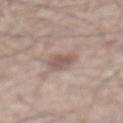notes = imaged on a skin check; not biopsied
image = total-body-photography crop, ~15 mm field of view
patient = male, about 65 years old
anatomic site = the abdomen